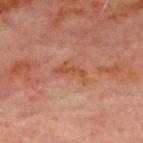Assessment: Recorded during total-body skin imaging; not selected for excision or biopsy. Background: The total-body-photography lesion software estimated a within-lesion color-variation index near 1.5/10 and peripheral color asymmetry of about 0.5. The subject is a male about 70 years old. Approximately 4 mm at its widest. The lesion is located on the chest. Cropped from a whole-body photographic skin survey; the tile spans about 15 mm. Captured under cross-polarized illumination.